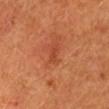This lesion was catalogued during total-body skin photography and was not selected for biopsy.
A region of skin cropped from a whole-body photographic capture, roughly 15 mm wide.
Located on the head or neck.
A patient aged 68–72.
Automated tile analysis of the lesion measured roughly 7 lightness units darker than nearby skin. And it measured an automated nevus-likeness rating near 15 out of 100 and a lesion-detection confidence of about 100/100.